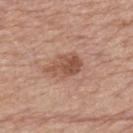Q: Was this lesion biopsied?
A: catalogued during a skin exam; not biopsied
Q: How large is the lesion?
A: ≈4.5 mm
Q: Who is the patient?
A: male, in their 80s
Q: Where on the body is the lesion?
A: the upper back
Q: What is the imaging modality?
A: ~15 mm tile from a whole-body skin photo
Q: What lighting was used for the tile?
A: white-light